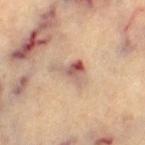On the left thigh. An algorithmic analysis of the crop reported a classifier nevus-likeness of about 0/100 and lesion-presence confidence of about 90/100. The patient is a female aged 63 to 67. A lesion tile, about 15 mm wide, cut from a 3D total-body photograph. This is a cross-polarized tile.